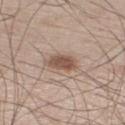Impression:
This lesion was catalogued during total-body skin photography and was not selected for biopsy.
Clinical summary:
A male patient approximately 60 years of age. The recorded lesion diameter is about 4 mm. A 15 mm close-up tile from a total-body photography series done for melanoma screening. The total-body-photography lesion software estimated a footprint of about 7 mm², an eccentricity of roughly 0.8, and two-axis asymmetry of about 0.25. The software also gave a mean CIELAB color near L≈53 a*≈17 b*≈26, about 12 CIELAB-L* units darker than the surrounding skin, and a lesion-to-skin contrast of about 8.5 (normalized; higher = more distinct). And it measured an automated nevus-likeness rating near 95 out of 100. Located on the right lower leg. This is a white-light tile.